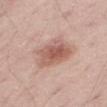biopsy status: no biopsy performed (imaged during a skin exam) | body site: the leg | patient: male, aged 48 to 52 | size: about 5.5 mm | acquisition: total-body-photography crop, ~15 mm field of view.